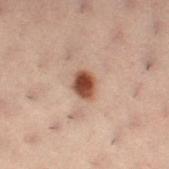Captured during whole-body skin photography for melanoma surveillance; the lesion was not biopsied. Located on the leg. The subject is a female aged 53–57. The lesion-visualizer software estimated a mean CIELAB color near L≈41 a*≈19 b*≈26, roughly 15 lightness units darker than nearby skin, and a normalized lesion–skin contrast near 12. It also reported radial color variation of about 1.5. It also reported a classifier nevus-likeness of about 100/100 and a lesion-detection confidence of about 100/100. A 15 mm close-up tile from a total-body photography series done for melanoma screening. About 3 mm across.